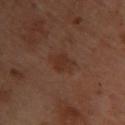A female subject in their mid- to late 50s. About 4 mm across. Automated tile analysis of the lesion measured a lesion color around L≈25 a*≈16 b*≈22 in CIELAB and a lesion-to-skin contrast of about 6 (normalized; higher = more distinct). A 15 mm crop from a total-body photograph taken for skin-cancer surveillance. On the chest.